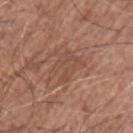The lesion is located on the arm. A male subject aged around 75. Cropped from a whole-body photographic skin survey; the tile spans about 15 mm.